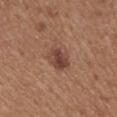biopsy status — catalogued during a skin exam; not biopsied
illumination — white-light illumination
lesion diameter — about 3 mm
location — the back
patient — male, aged around 65
image source — ~15 mm crop, total-body skin-cancer survey
image-analysis metrics — a lesion color around L≈44 a*≈21 b*≈26 in CIELAB and roughly 10 lightness units darker than nearby skin; a border-irregularity index near 2/10, internal color variation of about 4.5 on a 0–10 scale, and radial color variation of about 1.5